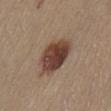Q: Is there a histopathology result?
A: total-body-photography surveillance lesion; no biopsy
Q: What is the imaging modality?
A: total-body-photography crop, ~15 mm field of view
Q: Lesion location?
A: the abdomen
Q: What is the lesion's diameter?
A: ≈5 mm
Q: How was the tile lit?
A: white-light
Q: What are the patient's age and sex?
A: female, aged 63–67